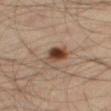The lesion was photographed on a routine skin check and not biopsied; there is no pathology result. A close-up tile cropped from a whole-body skin photograph, about 15 mm across. The lesion is located on the right thigh. The subject is a male aged 33 to 37.